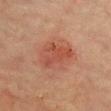Clinical impression: The lesion was tiled from a total-body skin photograph and was not biopsied. Context: The subject is a female aged 78–82. The lesion-visualizer software estimated a shape eccentricity near 0.7 and two-axis asymmetry of about 0.25. A 15 mm close-up tile from a total-body photography series done for melanoma screening. Approximately 4.5 mm at its widest. The lesion is located on the chest. This is a cross-polarized tile.